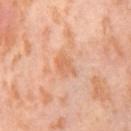Notes:
- follow-up — no biopsy performed (imaged during a skin exam)
- image source — ~15 mm tile from a whole-body skin photo
- subject — female, aged approximately 55
- lesion diameter — ~3 mm (longest diameter)
- location — the right thigh
- automated lesion analysis — a mean CIELAB color near L≈66 a*≈25 b*≈37; a border-irregularity index near 3.5/10, a within-lesion color-variation index near 2.5/10, and radial color variation of about 0.5